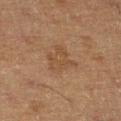Impression:
No biopsy was performed on this lesion — it was imaged during a full skin examination and was not determined to be concerning.
Acquisition and patient details:
The lesion is on the right lower leg. The patient is a male approximately 60 years of age. A lesion tile, about 15 mm wide, cut from a 3D total-body photograph.